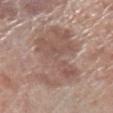{
  "biopsy_status": "not biopsied; imaged during a skin examination",
  "image": {
    "source": "total-body photography crop",
    "field_of_view_mm": 15
  },
  "site": "left lower leg",
  "patient": {
    "sex": "male",
    "age_approx": 70
  }
}